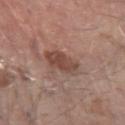• notes: imaged on a skin check; not biopsied
• anatomic site: the left forearm
• patient: male, aged 68–72
• image: ~15 mm crop, total-body skin-cancer survey